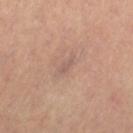biopsy status — no biopsy performed (imaged during a skin exam)
body site — the left thigh
lesion diameter — ≈2.5 mm
imaging modality — ~15 mm crop, total-body skin-cancer survey
subject — female, approximately 65 years of age
automated metrics — a shape eccentricity near 0.9 and a shape-asymmetry score of about 0.3 (0 = symmetric); a mean CIELAB color near L≈52 a*≈16 b*≈23, about 6 CIELAB-L* units darker than the surrounding skin, and a normalized border contrast of about 4.5; a detector confidence of about 75 out of 100 that the crop contains a lesion